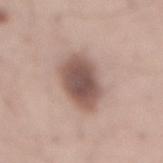This lesion was catalogued during total-body skin photography and was not selected for biopsy.
The patient is a male aged 53–57.
On the mid back.
Cropped from a whole-body photographic skin survey; the tile spans about 15 mm.
This is a white-light tile.
The recorded lesion diameter is about 6 mm.